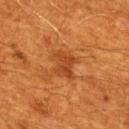The lesion was tiled from a total-body skin photograph and was not biopsied.
A male subject, aged 58 to 62.
A 15 mm crop from a total-body photograph taken for skin-cancer surveillance.
The lesion is located on the mid back.